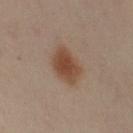Impression:
Captured during whole-body skin photography for melanoma surveillance; the lesion was not biopsied.
Context:
The subject is a female approximately 40 years of age. The lesion is located on the left arm. A 15 mm close-up tile from a total-body photography series done for melanoma screening. An algorithmic analysis of the crop reported an area of roughly 11 mm², an eccentricity of roughly 0.65, and a symmetry-axis asymmetry near 0.2. It also reported a lesion color around L≈46 a*≈19 b*≈29 in CIELAB and a normalized lesion–skin contrast near 9. It also reported border irregularity of about 2 on a 0–10 scale and radial color variation of about 0.5. Captured under cross-polarized illumination.